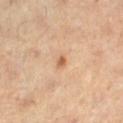  biopsy_status: not biopsied; imaged during a skin examination
  image:
    source: total-body photography crop
    field_of_view_mm: 15
  lighting: cross-polarized
  site: left lower leg
  lesion_size:
    long_diameter_mm_approx: 1.5
  patient:
    sex: female
    age_approx: 40